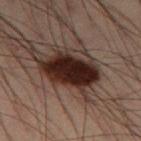Assessment: Imaged during a routine full-body skin examination; the lesion was not biopsied and no histopathology is available. Context: This image is a 15 mm lesion crop taken from a total-body photograph. The tile uses cross-polarized illumination. Located on the left thigh. The total-body-photography lesion software estimated roughly 17 lightness units darker than nearby skin and a normalized border contrast of about 19. And it measured a border-irregularity index near 2/10. A male patient, about 55 years old. The lesion's longest dimension is about 6 mm.